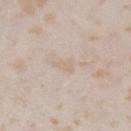Impression: No biopsy was performed on this lesion — it was imaged during a full skin examination and was not determined to be concerning. Image and clinical context: This image is a 15 mm lesion crop taken from a total-body photograph. On the left lower leg. An algorithmic analysis of the crop reported roughly 5 lightness units darker than nearby skin and a lesion-to-skin contrast of about 4.5 (normalized; higher = more distinct). The analysis additionally found a border-irregularity index near 3.5/10, a within-lesion color-variation index near 0/10, and peripheral color asymmetry of about 0. The subject is a female aged 23 to 27. The tile uses white-light illumination. Longest diameter approximately 2.5 mm.